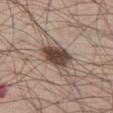Impression:
Captured during whole-body skin photography for melanoma surveillance; the lesion was not biopsied.
Clinical summary:
On the leg. A lesion tile, about 15 mm wide, cut from a 3D total-body photograph. A male patient aged approximately 55. The lesion's longest dimension is about 4 mm. Captured under white-light illumination.